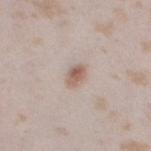Case summary:
• biopsy status — catalogued during a skin exam; not biopsied
• tile lighting — white-light illumination
• image source — total-body-photography crop, ~15 mm field of view
• body site — the left thigh
• patient — female, approximately 25 years of age